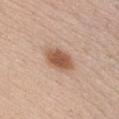Clinical impression: The lesion was photographed on a routine skin check and not biopsied; there is no pathology result. Context: The lesion is on the chest. A roughly 15 mm field-of-view crop from a total-body skin photograph. Longest diameter approximately 4 mm. The subject is a male aged around 35.